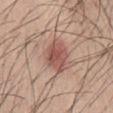Clinical impression: No biopsy was performed on this lesion — it was imaged during a full skin examination and was not determined to be concerning. Background: Cropped from a total-body skin-imaging series; the visible field is about 15 mm. A male subject aged 38–42. An algorithmic analysis of the crop reported an area of roughly 10 mm², an outline eccentricity of about 0.65 (0 = round, 1 = elongated), and two-axis asymmetry of about 0.2. It also reported a border-irregularity index near 2/10, a color-variation rating of about 4.5/10, and a peripheral color-asymmetry measure near 1.5. And it measured an automated nevus-likeness rating near 90 out of 100. Captured under white-light illumination. The lesion is on the abdomen. About 4 mm across.